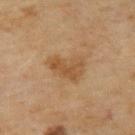<case>
<biopsy_status>not biopsied; imaged during a skin examination</biopsy_status>
<image>
  <source>total-body photography crop</source>
  <field_of_view_mm>15</field_of_view_mm>
</image>
<site>upper back</site>
<patient>
  <sex>female</sex>
  <age_approx>60</age_approx>
</patient>
<lighting>cross-polarized</lighting>
<lesion_size>
  <long_diameter_mm_approx>4.0</long_diameter_mm_approx>
</lesion_size>
<automated_metrics>
  <area_mm2_approx>9.5</area_mm2_approx>
  <eccentricity>0.7</eccentricity>
  <shape_asymmetry>0.4</shape_asymmetry>
  <cielab_L>47</cielab_L>
  <cielab_a>17</cielab_a>
  <cielab_b>34</cielab_b>
  <vs_skin_contrast_norm>6.5</vs_skin_contrast_norm>
  <border_irregularity_0_10>4.5</border_irregularity_0_10>
  <peripheral_color_asymmetry>1.0</peripheral_color_asymmetry>
  <nevus_likeness_0_100>35</nevus_likeness_0_100>
  <lesion_detection_confidence_0_100>100</lesion_detection_confidence_0_100>
</automated_metrics>
</case>